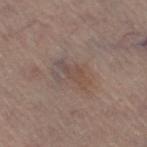notes: total-body-photography surveillance lesion; no biopsy | diameter: about 4 mm | subject: female, aged 63 to 67 | image: ~15 mm crop, total-body skin-cancer survey | anatomic site: the right thigh.